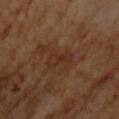Imaged during a routine full-body skin examination; the lesion was not biopsied and no histopathology is available. Automated tile analysis of the lesion measured an area of roughly 4.5 mm², a shape eccentricity near 0.95, and a symmetry-axis asymmetry near 0.3. A region of skin cropped from a whole-body photographic capture, roughly 15 mm wide. A male patient approximately 65 years of age. The recorded lesion diameter is about 3.5 mm. This is a cross-polarized tile.